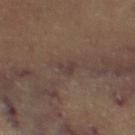Clinical impression:
No biopsy was performed on this lesion — it was imaged during a full skin examination and was not determined to be concerning.
Context:
A 15 mm crop from a total-body photograph taken for skin-cancer surveillance. A subject aged 58 to 62. Located on the leg.